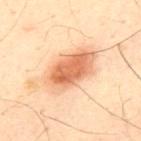Assessment: No biopsy was performed on this lesion — it was imaged during a full skin examination and was not determined to be concerning. Acquisition and patient details: A 15 mm close-up extracted from a 3D total-body photography capture. The lesion is located on the upper back. A male subject, roughly 50 years of age.